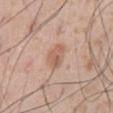notes: imaged on a skin check; not biopsied | tile lighting: white-light | subject: male, in their mid- to late 50s | imaging modality: ~15 mm crop, total-body skin-cancer survey | location: the abdomen | size: about 3.5 mm.